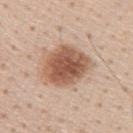This lesion was catalogued during total-body skin photography and was not selected for biopsy. Located on the back. A close-up tile cropped from a whole-body skin photograph, about 15 mm across. Longest diameter approximately 5.5 mm. Automated tile analysis of the lesion measured an area of roughly 20 mm² and an eccentricity of roughly 0.55. It also reported a mean CIELAB color near L≈56 a*≈21 b*≈30, roughly 15 lightness units darker than nearby skin, and a normalized lesion–skin contrast near 10. A male patient approximately 60 years of age. The tile uses white-light illumination.